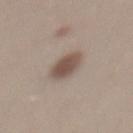The lesion was photographed on a routine skin check and not biopsied; there is no pathology result. The patient is a male in their 30s. A 15 mm close-up extracted from a 3D total-body photography capture. The recorded lesion diameter is about 3.5 mm. The lesion is located on the mid back. Imaged with white-light lighting. The total-body-photography lesion software estimated a lesion color around L≈51 a*≈14 b*≈23 in CIELAB, about 12 CIELAB-L* units darker than the surrounding skin, and a lesion-to-skin contrast of about 9 (normalized; higher = more distinct). It also reported a nevus-likeness score of about 100/100 and a detector confidence of about 100 out of 100 that the crop contains a lesion.